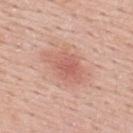| field | value |
|---|---|
| workup | catalogued during a skin exam; not biopsied |
| body site | the upper back |
| lesion diameter | ≈5 mm |
| image | 15 mm crop, total-body photography |
| patient | male, about 55 years old |